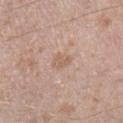Case summary:
• follow-up: no biopsy performed (imaged during a skin exam)
• lesion diameter: ≈2.5 mm
• image source: ~15 mm tile from a whole-body skin photo
• illumination: white-light
• patient: male, aged around 45
• location: the right lower leg